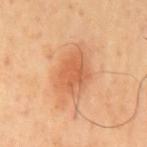biopsy_status: not biopsied; imaged during a skin examination
image:
  source: total-body photography crop
  field_of_view_mm: 15
site: mid back
patient:
  sex: male
  age_approx: 65